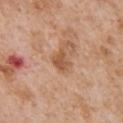Q: Was a biopsy performed?
A: no biopsy performed (imaged during a skin exam)
Q: Lesion location?
A: the chest
Q: How was the tile lit?
A: white-light
Q: What is the imaging modality?
A: ~15 mm crop, total-body skin-cancer survey
Q: Who is the patient?
A: male, about 65 years old